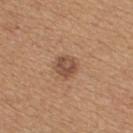<tbp_lesion>
  <site>upper back</site>
  <lighting>white-light</lighting>
  <lesion_size>
    <long_diameter_mm_approx>2.5</long_diameter_mm_approx>
  </lesion_size>
  <patient>
    <sex>female</sex>
    <age_approx>45</age_approx>
  </patient>
  <image>
    <source>total-body photography crop</source>
    <field_of_view_mm>15</field_of_view_mm>
  </image>
</tbp_lesion>